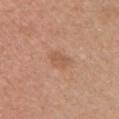Part of a total-body skin-imaging series; this lesion was reviewed on a skin check and was not flagged for biopsy.
A 15 mm close-up extracted from a 3D total-body photography capture.
Automated image analysis of the tile measured an automated nevus-likeness rating near 15 out of 100 and a detector confidence of about 100 out of 100 that the crop contains a lesion.
On the chest.
This is a white-light tile.
A female patient approximately 40 years of age.